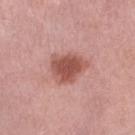Impression: The lesion was tiled from a total-body skin photograph and was not biopsied. Background: The subject is a female aged around 70. The lesion is located on the right lower leg. A roughly 15 mm field-of-view crop from a total-body skin photograph. The lesion's longest dimension is about 4.5 mm. The total-body-photography lesion software estimated a lesion area of about 11 mm² and a symmetry-axis asymmetry near 0.25. It also reported a mean CIELAB color near L≈53 a*≈26 b*≈26, a lesion–skin lightness drop of about 13, and a normalized lesion–skin contrast near 9. The software also gave a classifier nevus-likeness of about 90/100 and a detector confidence of about 100 out of 100 that the crop contains a lesion.